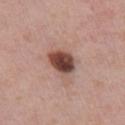• workup — catalogued during a skin exam; not biopsied
• image source — total-body-photography crop, ~15 mm field of view
• image-analysis metrics — a lesion area of about 9 mm², an eccentricity of roughly 0.65, and a shape-asymmetry score of about 0.2 (0 = symmetric); a lesion–skin lightness drop of about 18 and a normalized lesion–skin contrast near 12.5
• illumination — white-light
• location — the chest
• diameter — ~4 mm (longest diameter)
• subject — male, roughly 60 years of age